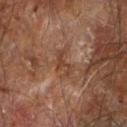Part of a total-body skin-imaging series; this lesion was reviewed on a skin check and was not flagged for biopsy. The patient is a male about 65 years old. A close-up tile cropped from a whole-body skin photograph, about 15 mm across. Located on the right forearm.